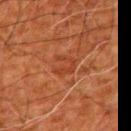The lesion was photographed on a routine skin check and not biopsied; there is no pathology result. This is a cross-polarized tile. Located on the left upper arm. A male patient aged 78–82. An algorithmic analysis of the crop reported a mean CIELAB color near L≈33 a*≈25 b*≈31, a lesion–skin lightness drop of about 5, and a normalized border contrast of about 5. A 15 mm close-up tile from a total-body photography series done for melanoma screening. Longest diameter approximately 2.5 mm.